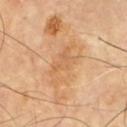<case>
<biopsy_status>not biopsied; imaged during a skin examination</biopsy_status>
<site>chest</site>
<lesion_size>
  <long_diameter_mm_approx>6.0</long_diameter_mm_approx>
</lesion_size>
<automated_metrics>
  <area_mm2_approx>9.0</area_mm2_approx>
  <eccentricity>0.9</eccentricity>
  <shape_asymmetry>0.45</shape_asymmetry>
  <nevus_likeness_0_100>0</nevus_likeness_0_100>
  <lesion_detection_confidence_0_100>100</lesion_detection_confidence_0_100>
</automated_metrics>
<image>
  <source>total-body photography crop</source>
  <field_of_view_mm>15</field_of_view_mm>
</image>
</case>